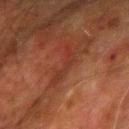follow-up = total-body-photography surveillance lesion; no biopsy | acquisition = total-body-photography crop, ~15 mm field of view | subject = male, aged 78–82 | lighting = cross-polarized illumination | lesion diameter = ~4.5 mm (longest diameter) | image-analysis metrics = roughly 5 lightness units darker than nearby skin and a normalized border contrast of about 5; border irregularity of about 8.5 on a 0–10 scale, a within-lesion color-variation index near 0.5/10, and peripheral color asymmetry of about 0; a nevus-likeness score of about 0/100 and a lesion-detection confidence of about 50/100 | body site = the left upper arm.